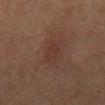Assessment:
This lesion was catalogued during total-body skin photography and was not selected for biopsy.
Background:
This is a cross-polarized tile. The lesion is on the mid back. A roughly 15 mm field-of-view crop from a total-body skin photograph. Automated image analysis of the tile measured a footprint of about 3.5 mm², an eccentricity of roughly 0.8, and two-axis asymmetry of about 0.35. The analysis additionally found a lesion color around L≈32 a*≈19 b*≈22 in CIELAB and a lesion-to-skin contrast of about 4.5 (normalized; higher = more distinct). And it measured a border-irregularity rating of about 3.5/10, internal color variation of about 1.5 on a 0–10 scale, and peripheral color asymmetry of about 0.5. It also reported an automated nevus-likeness rating near 5 out of 100 and a lesion-detection confidence of about 100/100. The lesion's longest dimension is about 2.5 mm. A male patient about 70 years old.